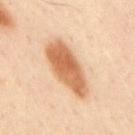biopsy_status: not biopsied; imaged during a skin examination
site: mid back
image:
  source: total-body photography crop
  field_of_view_mm: 15
patient:
  sex: male
  age_approx: 45
automated_metrics:
  area_mm2_approx: 20.0
  cielab_L: 64
  cielab_a: 22
  cielab_b: 38
  vs_skin_contrast_norm: 9.5
  border_irregularity_0_10: 2.5
  color_variation_0_10: 4.5
  peripheral_color_asymmetry: 1.5
lesion_size:
  long_diameter_mm_approx: 7.5
lighting: cross-polarized